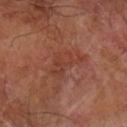{"biopsy_status": "not biopsied; imaged during a skin examination", "lighting": "cross-polarized", "lesion_size": {"long_diameter_mm_approx": 4.5}, "site": "arm", "automated_metrics": {"cielab_L": 38, "cielab_a": 24, "cielab_b": 28, "vs_skin_darker_L": 6.0, "vs_skin_contrast_norm": 5.0, "nevus_likeness_0_100": 0, "lesion_detection_confidence_0_100": 100}, "image": {"source": "total-body photography crop", "field_of_view_mm": 15}, "patient": {"sex": "male", "age_approx": 70}}